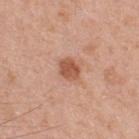Assessment:
No biopsy was performed on this lesion — it was imaged during a full skin examination and was not determined to be concerning.
Context:
Captured under white-light illumination. A male patient, approximately 55 years of age. The lesion is on the chest. An algorithmic analysis of the crop reported a lesion area of about 5.5 mm², a shape eccentricity near 0.6, and a shape-asymmetry score of about 0.25 (0 = symmetric). The software also gave border irregularity of about 2 on a 0–10 scale and peripheral color asymmetry of about 1. And it measured an automated nevus-likeness rating near 85 out of 100 and lesion-presence confidence of about 100/100. Approximately 3 mm at its widest. Cropped from a total-body skin-imaging series; the visible field is about 15 mm.